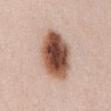Assessment:
Captured during whole-body skin photography for melanoma surveillance; the lesion was not biopsied.
Context:
The recorded lesion diameter is about 6.5 mm. The lesion-visualizer software estimated a mean CIELAB color near L≈52 a*≈21 b*≈28, a lesion–skin lightness drop of about 21, and a lesion-to-skin contrast of about 13.5 (normalized; higher = more distinct). The software also gave a classifier nevus-likeness of about 100/100 and lesion-presence confidence of about 100/100. Imaged with white-light lighting. The lesion is located on the abdomen. A female patient, aged around 40. Cropped from a total-body skin-imaging series; the visible field is about 15 mm.